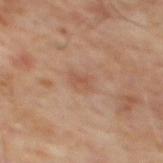<record>
<biopsy_status>not biopsied; imaged during a skin examination</biopsy_status>
<lighting>cross-polarized</lighting>
<patient>
  <sex>male</sex>
  <age_approx>65</age_approx>
</patient>
<lesion_size>
  <long_diameter_mm_approx>2.5</long_diameter_mm_approx>
</lesion_size>
<site>back</site>
<image>
  <source>total-body photography crop</source>
  <field_of_view_mm>15</field_of_view_mm>
</image>
<automated_metrics>
  <cielab_L>51</cielab_L>
  <cielab_a>20</cielab_a>
  <cielab_b>29</cielab_b>
  <vs_skin_darker_L>6.0</vs_skin_darker_L>
  <vs_skin_contrast_norm>5.0</vs_skin_contrast_norm>
  <nevus_likeness_0_100>0</nevus_likeness_0_100>
  <lesion_detection_confidence_0_100>100</lesion_detection_confidence_0_100>
</automated_metrics>
</record>